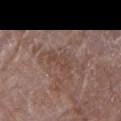{"biopsy_status": "not biopsied; imaged during a skin examination", "image": {"source": "total-body photography crop", "field_of_view_mm": 15}, "lighting": "white-light", "patient": {"sex": "female", "age_approx": 70}, "automated_metrics": {"border_irregularity_0_10": 5.0, "color_variation_0_10": 1.5, "peripheral_color_asymmetry": 0.5, "nevus_likeness_0_100": 0}, "site": "right upper arm", "lesion_size": {"long_diameter_mm_approx": 3.5}}